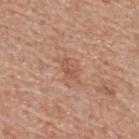Clinical impression:
The lesion was photographed on a routine skin check and not biopsied; there is no pathology result.
Clinical summary:
A male patient, aged approximately 55. From the upper back. A 15 mm close-up tile from a total-body photography series done for melanoma screening. Imaged with white-light lighting. The lesion's longest dimension is about 3 mm.